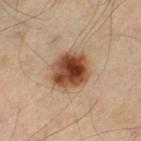Findings:
– follow-up · total-body-photography surveillance lesion; no biopsy